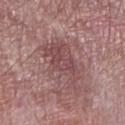Notes:
* biopsy status · imaged on a skin check; not biopsied
* subject · male, aged 68 to 72
* location · the right lower leg
* TBP lesion metrics · a footprint of about 16 mm², an eccentricity of roughly 0.9, and a symmetry-axis asymmetry near 0.45; an average lesion color of about L≈47 a*≈22 b*≈19 (CIELAB) and a normalized lesion–skin contrast near 6.5; a border-irregularity rating of about 7/10, internal color variation of about 3.5 on a 0–10 scale, and radial color variation of about 1.5; a nevus-likeness score of about 0/100 and a lesion-detection confidence of about 90/100
* acquisition · ~15 mm crop, total-body skin-cancer survey
* size · ~7 mm (longest diameter)